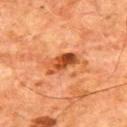{"biopsy_status": "not biopsied; imaged during a skin examination", "image": {"source": "total-body photography crop", "field_of_view_mm": 15}, "patient": {"sex": "male", "age_approx": 65}, "automated_metrics": {"area_mm2_approx": 9.0, "eccentricity": 0.9, "shape_asymmetry": 0.35, "border_irregularity_0_10": 4.5, "color_variation_0_10": 8.5, "peripheral_color_asymmetry": 3.0}, "site": "back"}